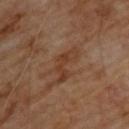Captured during whole-body skin photography for melanoma surveillance; the lesion was not biopsied. From the upper back. A lesion tile, about 15 mm wide, cut from a 3D total-body photograph. The subject is a male roughly 70 years of age. The recorded lesion diameter is about 4.5 mm. The tile uses cross-polarized illumination.